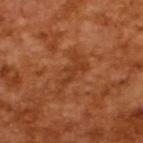patient=male, in their mid- to late 60s | imaging modality=total-body-photography crop, ~15 mm field of view.